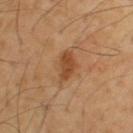Assessment:
Imaged during a routine full-body skin examination; the lesion was not biopsied and no histopathology is available.
Context:
A close-up tile cropped from a whole-body skin photograph, about 15 mm across. The tile uses cross-polarized illumination. From the upper back. A male patient, aged 53–57. Longest diameter approximately 3.5 mm.